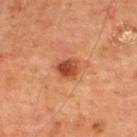biopsy status = catalogued during a skin exam; not biopsied | illumination = cross-polarized | automated lesion analysis = an average lesion color of about L≈49 a*≈30 b*≈38 (CIELAB), roughly 13 lightness units darker than nearby skin, and a normalized border contrast of about 9; border irregularity of about 2 on a 0–10 scale, a within-lesion color-variation index near 6/10, and peripheral color asymmetry of about 2; a classifier nevus-likeness of about 90/100 | imaging modality = ~15 mm tile from a whole-body skin photo | site = the upper back | subject = male, in their mid- to late 60s | lesion size = ≈3 mm.